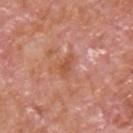{"lesion_size": {"long_diameter_mm_approx": 3.0}, "lighting": "white-light", "image": {"source": "total-body photography crop", "field_of_view_mm": 15}, "site": "upper back", "patient": {"sex": "male", "age_approx": 65}}